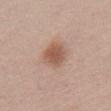Case summary:
– follow-up: total-body-photography surveillance lesion; no biopsy
– image source: ~15 mm crop, total-body skin-cancer survey
– lesion diameter: ≈3.5 mm
– automated metrics: a footprint of about 9 mm², an outline eccentricity of about 0.6 (0 = round, 1 = elongated), and two-axis asymmetry of about 0.15; a normalized lesion–skin contrast near 7.5
– site: the chest
– patient: female, approximately 50 years of age